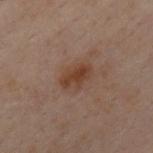<lesion>
  <biopsy_status>not biopsied; imaged during a skin examination</biopsy_status>
  <automated_metrics>
    <color_variation_0_10>3.0</color_variation_0_10>
    <peripheral_color_asymmetry>1.0</peripheral_color_asymmetry>
  </automated_metrics>
  <image>
    <source>total-body photography crop</source>
    <field_of_view_mm>15</field_of_view_mm>
  </image>
  <patient>
    <sex>male</sex>
    <age_approx>30</age_approx>
  </patient>
  <site>chest</site>
  <lighting>cross-polarized</lighting>
  <lesion_size>
    <long_diameter_mm_approx>3.5</long_diameter_mm_approx>
  </lesion_size>
</lesion>